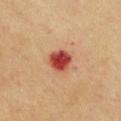Recorded during total-body skin imaging; not selected for excision or biopsy.
A lesion tile, about 15 mm wide, cut from a 3D total-body photograph.
Approximately 3 mm at its widest.
The subject is a female approximately 55 years of age.
Located on the front of the torso.
Automated image analysis of the tile measured an average lesion color of about L≈41 a*≈32 b*≈29 (CIELAB), roughly 16 lightness units darker than nearby skin, and a normalized border contrast of about 12. The software also gave a border-irregularity rating of about 1.5/10, internal color variation of about 5.5 on a 0–10 scale, and peripheral color asymmetry of about 1.5. The software also gave a nevus-likeness score of about 0/100.
Imaged with cross-polarized lighting.